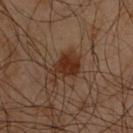  biopsy_status: not biopsied; imaged during a skin examination
  automated_metrics:
    area_mm2_approx: 8.0
    eccentricity: 0.7
    cielab_L: 27
    cielab_a: 18
    cielab_b: 26
    vs_skin_darker_L: 9.0
    vs_skin_contrast_norm: 9.5
    border_irregularity_0_10: 4.5
    peripheral_color_asymmetry: 1.0
    nevus_likeness_0_100: 90
    lesion_detection_confidence_0_100: 100
  site: chest
  lesion_size:
    long_diameter_mm_approx: 4.0
  patient:
    sex: male
    age_approx: 60
  image:
    source: total-body photography crop
    field_of_view_mm: 15
  lighting: cross-polarized